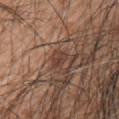No biopsy was performed on this lesion — it was imaged during a full skin examination and was not determined to be concerning. The total-body-photography lesion software estimated a border-irregularity index near 3.5/10, a within-lesion color-variation index near 0/10, and radial color variation of about 0. It also reported a classifier nevus-likeness of about 15/100 and a detector confidence of about 55 out of 100 that the crop contains a lesion. The lesion's longest dimension is about 2.5 mm. From the upper back. A close-up tile cropped from a whole-body skin photograph, about 15 mm across. This is a white-light tile. The subject is a male aged approximately 50.